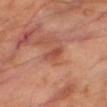Q: What kind of image is this?
A: ~15 mm crop, total-body skin-cancer survey
Q: Automated lesion metrics?
A: a mean CIELAB color near L≈48 a*≈27 b*≈31 and a lesion-to-skin contrast of about 6 (normalized; higher = more distinct); a color-variation rating of about 1/10
Q: Lesion location?
A: the right thigh
Q: Patient demographics?
A: male, roughly 70 years of age
Q: What is the lesion's diameter?
A: ≈2.5 mm